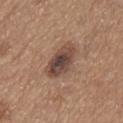image source: 15 mm crop, total-body photography | subject: male, in their mid- to late 60s | automated lesion analysis: an area of roughly 10 mm², an eccentricity of roughly 0.85, and two-axis asymmetry of about 0.25; a lesion-detection confidence of about 100/100 | anatomic site: the abdomen | tile lighting: white-light illumination | lesion size: ≈5 mm.